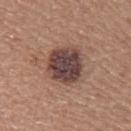biopsy_status: not biopsied; imaged during a skin examination
site: right upper arm
patient:
  sex: female
  age_approx: 65
image:
  source: total-body photography crop
  field_of_view_mm: 15